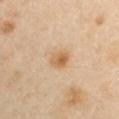follow-up: imaged on a skin check; not biopsied | automated lesion analysis: an area of roughly 5.5 mm², an eccentricity of roughly 0.55, and a shape-asymmetry score of about 0.15 (0 = symmetric); an average lesion color of about L≈61 a*≈17 b*≈37 (CIELAB) and a normalized border contrast of about 7.5; internal color variation of about 5.5 on a 0–10 scale and radial color variation of about 1.5 | patient: male, in their 40s | illumination: cross-polarized | image: total-body-photography crop, ~15 mm field of view | diameter: ≈2.5 mm | location: the right upper arm.